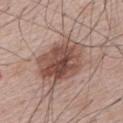This lesion was catalogued during total-body skin photography and was not selected for biopsy. Cropped from a whole-body photographic skin survey; the tile spans about 15 mm. On the upper back. A male patient about 65 years old. The tile uses white-light illumination. Measured at roughly 7 mm in maximum diameter.